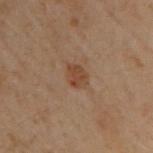<lesion>
  <biopsy_status>not biopsied; imaged during a skin examination</biopsy_status>
  <site>back</site>
  <lighting>cross-polarized</lighting>
  <image>
    <source>total-body photography crop</source>
    <field_of_view_mm>15</field_of_view_mm>
  </image>
  <patient>
    <sex>male</sex>
    <age_approx>50</age_approx>
  </patient>
  <lesion_size>
    <long_diameter_mm_approx>3.0</long_diameter_mm_approx>
  </lesion_size>
</lesion>